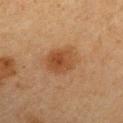This lesion was catalogued during total-body skin photography and was not selected for biopsy. The patient is a male about 65 years old. From the right upper arm. The tile uses cross-polarized illumination. A close-up tile cropped from a whole-body skin photograph, about 15 mm across.